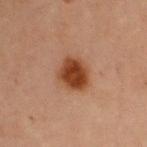{"biopsy_status": "not biopsied; imaged during a skin examination", "lighting": "cross-polarized", "lesion_size": {"long_diameter_mm_approx": 4.0}, "image": {"source": "total-body photography crop", "field_of_view_mm": 15}, "patient": {"sex": "female", "age_approx": 70}, "automated_metrics": {"cielab_L": 38, "cielab_a": 22, "cielab_b": 31, "vs_skin_darker_L": 12.0, "vs_skin_contrast_norm": 11.0, "lesion_detection_confidence_0_100": 100}, "site": "right upper arm"}